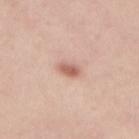Notes:
* biopsy status: total-body-photography surveillance lesion; no biopsy
* TBP lesion metrics: an automated nevus-likeness rating near 95 out of 100 and a lesion-detection confidence of about 100/100
* image source: ~15 mm tile from a whole-body skin photo
* lighting: white-light
* subject: female, aged 28 to 32
* site: the mid back